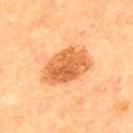Recorded during total-body skin imaging; not selected for excision or biopsy.
A female subject roughly 55 years of age.
From the upper back.
A region of skin cropped from a whole-body photographic capture, roughly 15 mm wide.
Longest diameter approximately 6 mm.